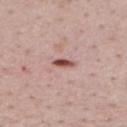follow-up: total-body-photography surveillance lesion; no biopsy | imaging modality: total-body-photography crop, ~15 mm field of view | patient: male, aged 48 to 52 | anatomic site: the mid back.